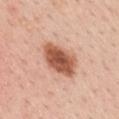<record>
<biopsy_status>not biopsied; imaged during a skin examination</biopsy_status>
<lesion_size>
  <long_diameter_mm_approx>5.0</long_diameter_mm_approx>
</lesion_size>
<image>
  <source>total-body photography crop</source>
  <field_of_view_mm>15</field_of_view_mm>
</image>
<lighting>white-light</lighting>
<patient>
  <sex>female</sex>
  <age_approx>40</age_approx>
</patient>
<automated_metrics>
  <area_mm2_approx>13.0</area_mm2_approx>
  <eccentricity>0.8</eccentricity>
  <shape_asymmetry>0.2</shape_asymmetry>
  <nevus_likeness_0_100>100</nevus_likeness_0_100>
  <lesion_detection_confidence_0_100>100</lesion_detection_confidence_0_100>
</automated_metrics>
<site>mid back</site>
</record>